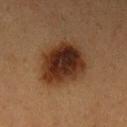Clinical impression:
This lesion was catalogued during total-body skin photography and was not selected for biopsy.
Context:
A female subject, aged approximately 40. A 15 mm close-up tile from a total-body photography series done for melanoma screening. The lesion is on the left upper arm.